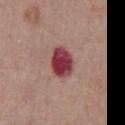workup: catalogued during a skin exam; not biopsied
tile lighting: white-light
location: the chest
lesion diameter: ~4 mm (longest diameter)
image source: total-body-photography crop, ~15 mm field of view
subject: male, aged approximately 55